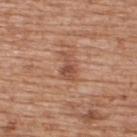patient:
  sex: male
  age_approx: 60
image:
  source: total-body photography crop
  field_of_view_mm: 15
lighting: white-light
site: upper back